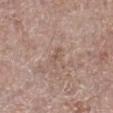Recorded during total-body skin imaging; not selected for excision or biopsy. A male subject roughly 55 years of age. This is a white-light tile. This image is a 15 mm lesion crop taken from a total-body photograph. The recorded lesion diameter is about 2.5 mm. The lesion is on the right lower leg.